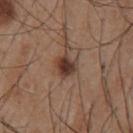site = the chest | image source = 15 mm crop, total-body photography | patient = male, in their mid-50s.